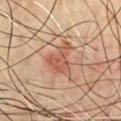Recorded during total-body skin imaging; not selected for excision or biopsy. An algorithmic analysis of the crop reported a lesion area of about 9.5 mm², an eccentricity of roughly 0.65, and a shape-asymmetry score of about 0.5 (0 = symmetric). It also reported a mean CIELAB color near L≈53 a*≈23 b*≈31, a lesion–skin lightness drop of about 9, and a normalized border contrast of about 6.5. The analysis additionally found border irregularity of about 5.5 on a 0–10 scale, a color-variation rating of about 3/10, and peripheral color asymmetry of about 1. The analysis additionally found a classifier nevus-likeness of about 10/100 and lesion-presence confidence of about 100/100. Located on the chest. A roughly 15 mm field-of-view crop from a total-body skin photograph. Longest diameter approximately 4.5 mm. The patient is a male about 70 years old. This is a cross-polarized tile.